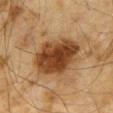Case summary:
– workup · catalogued during a skin exam; not biopsied
– patient · male, aged around 65
– image-analysis metrics · a lesion area of about 24 mm², an eccentricity of roughly 0.55, and two-axis asymmetry of about 0.15; roughly 16 lightness units darker than nearby skin; border irregularity of about 1.5 on a 0–10 scale and a within-lesion color-variation index near 5.5/10; a classifier nevus-likeness of about 100/100 and a detector confidence of about 100 out of 100 that the crop contains a lesion
– size · ≈6 mm
– anatomic site · the mid back
– acquisition · 15 mm crop, total-body photography
– lighting · cross-polarized illumination